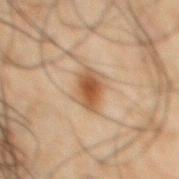<case>
<biopsy_status>not biopsied; imaged during a skin examination</biopsy_status>
<image>
  <source>total-body photography crop</source>
  <field_of_view_mm>15</field_of_view_mm>
</image>
<lighting>cross-polarized</lighting>
<site>chest</site>
<patient>
  <sex>male</sex>
  <age_approx>50</age_approx>
</patient>
<lesion_size>
  <long_diameter_mm_approx>3.5</long_diameter_mm_approx>
</lesion_size>
</case>